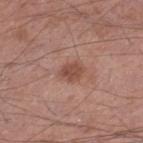This lesion was catalogued during total-body skin photography and was not selected for biopsy.
On the left thigh.
Longest diameter approximately 2.5 mm.
The tile uses white-light illumination.
An algorithmic analysis of the crop reported a lesion area of about 4.5 mm² and a shape-asymmetry score of about 0.3 (0 = symmetric). The analysis additionally found a mean CIELAB color near L≈48 a*≈23 b*≈27, roughly 10 lightness units darker than nearby skin, and a lesion-to-skin contrast of about 7.5 (normalized; higher = more distinct).
A 15 mm close-up extracted from a 3D total-body photography capture.
A male patient about 55 years old.